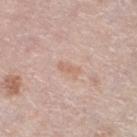Q: Is there a histopathology result?
A: no biopsy performed (imaged during a skin exam)
Q: What is the lesion's diameter?
A: about 2.5 mm
Q: What kind of image is this?
A: ~15 mm tile from a whole-body skin photo
Q: Patient demographics?
A: female, in their mid- to late 60s
Q: Where on the body is the lesion?
A: the left lower leg